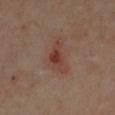Q: Is there a histopathology result?
A: no biopsy performed (imaged during a skin exam)
Q: What kind of image is this?
A: ~15 mm crop, total-body skin-cancer survey
Q: What are the patient's age and sex?
A: male, aged 63–67
Q: What lighting was used for the tile?
A: cross-polarized
Q: Lesion location?
A: the left lower leg